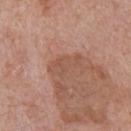Recorded during total-body skin imaging; not selected for excision or biopsy. A male subject aged around 70. A 15 mm close-up extracted from a 3D total-body photography capture. The lesion is on the front of the torso.